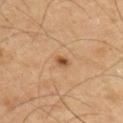Q: Was a biopsy performed?
A: catalogued during a skin exam; not biopsied
Q: Lesion location?
A: the arm
Q: Who is the patient?
A: male, aged 43 to 47
Q: What is the imaging modality?
A: ~15 mm tile from a whole-body skin photo
Q: What lighting was used for the tile?
A: cross-polarized illumination
Q: What is the lesion's diameter?
A: ~1.5 mm (longest diameter)